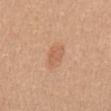Imaged during a routine full-body skin examination; the lesion was not biopsied and no histopathology is available.